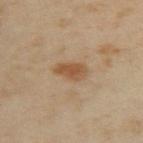workup: imaged on a skin check; not biopsied
TBP lesion metrics: an outline eccentricity of about 0.85 (0 = round, 1 = elongated) and a shape-asymmetry score of about 0.2 (0 = symmetric); border irregularity of about 2 on a 0–10 scale, a within-lesion color-variation index near 3/10, and a peripheral color-asymmetry measure near 1
tile lighting: cross-polarized illumination
anatomic site: the upper back
subject: female, aged approximately 35
lesion diameter: ≈3.5 mm
imaging modality: total-body-photography crop, ~15 mm field of view